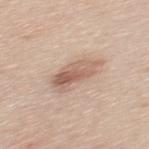| field | value |
|---|---|
| workup | imaged on a skin check; not biopsied |
| subject | male, roughly 35 years of age |
| imaging modality | ~15 mm tile from a whole-body skin photo |
| body site | the mid back |